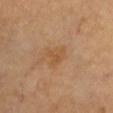Impression: No biopsy was performed on this lesion — it was imaged during a full skin examination and was not determined to be concerning. Image and clinical context: Located on the chest. About 2.5 mm across. Captured under cross-polarized illumination. A female subject aged 63 to 67. An algorithmic analysis of the crop reported a mean CIELAB color near L≈52 a*≈20 b*≈37, a lesion–skin lightness drop of about 6, and a lesion-to-skin contrast of about 5.5 (normalized; higher = more distinct). And it measured border irregularity of about 4.5 on a 0–10 scale, a color-variation rating of about 1.5/10, and radial color variation of about 0.5. Cropped from a whole-body photographic skin survey; the tile spans about 15 mm.